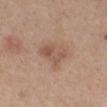Impression: Captured during whole-body skin photography for melanoma surveillance; the lesion was not biopsied.